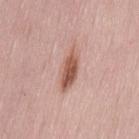No biopsy was performed on this lesion — it was imaged during a full skin examination and was not determined to be concerning.
Cropped from a total-body skin-imaging series; the visible field is about 15 mm.
On the lower back.
A female subject approximately 50 years of age.